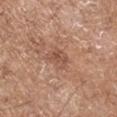| feature | finding |
|---|---|
| notes | total-body-photography surveillance lesion; no biopsy |
| size | about 3 mm |
| tile lighting | white-light illumination |
| TBP lesion metrics | a footprint of about 4.5 mm², an outline eccentricity of about 0.8 (0 = round, 1 = elongated), and a symmetry-axis asymmetry near 0.25; a mean CIELAB color near L≈52 a*≈22 b*≈29 and roughly 8 lightness units darker than nearby skin |
| acquisition | ~15 mm tile from a whole-body skin photo |
| subject | male, approximately 70 years of age |
| location | the left upper arm |